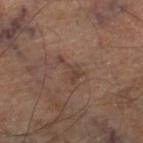The lesion was photographed on a routine skin check and not biopsied; there is no pathology result.
Cropped from a total-body skin-imaging series; the visible field is about 15 mm.
Approximately 3.5 mm at its widest.
From the right thigh.